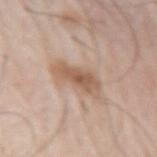Q: Was this lesion biopsied?
A: imaged on a skin check; not biopsied
Q: Automated lesion metrics?
A: a lesion color around L≈58 a*≈18 b*≈29 in CIELAB, a lesion–skin lightness drop of about 11, and a lesion-to-skin contrast of about 7.5 (normalized; higher = more distinct); border irregularity of about 3.5 on a 0–10 scale and a within-lesion color-variation index near 3/10; a nevus-likeness score of about 85/100
Q: Patient demographics?
A: male, aged around 80
Q: Lesion location?
A: the arm
Q: What kind of image is this?
A: ~15 mm crop, total-body skin-cancer survey
Q: Lesion size?
A: ~5 mm (longest diameter)